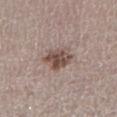Impression: No biopsy was performed on this lesion — it was imaged during a full skin examination and was not determined to be concerning.